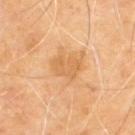{
  "biopsy_status": "not biopsied; imaged during a skin examination",
  "image": {
    "source": "total-body photography crop",
    "field_of_view_mm": 15
  },
  "patient": {
    "sex": "male",
    "age_approx": 70
  },
  "lighting": "cross-polarized",
  "lesion_size": {
    "long_diameter_mm_approx": 3.0
  },
  "site": "upper back"
}